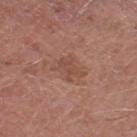The lesion was photographed on a routine skin check and not biopsied; there is no pathology result. Automated tile analysis of the lesion measured a lesion area of about 7 mm², a shape eccentricity near 0.75, and a shape-asymmetry score of about 0.35 (0 = symmetric). It also reported an average lesion color of about L≈49 a*≈22 b*≈28 (CIELAB), about 7 CIELAB-L* units darker than the surrounding skin, and a normalized lesion–skin contrast near 5. This image is a 15 mm lesion crop taken from a total-body photograph. The lesion is on the left thigh. Measured at roughly 3.5 mm in maximum diameter. A male subject, in their mid- to late 60s.